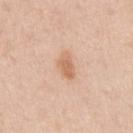Imaged during a routine full-body skin examination; the lesion was not biopsied and no histopathology is available. A male subject, in their 50s. Captured under white-light illumination. A lesion tile, about 15 mm wide, cut from a 3D total-body photograph. Located on the right upper arm. About 3 mm across. Automated image analysis of the tile measured a border-irregularity rating of about 3/10 and a peripheral color-asymmetry measure near 0.5. And it measured an automated nevus-likeness rating near 80 out of 100 and lesion-presence confidence of about 100/100.